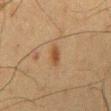Assessment: The lesion was photographed on a routine skin check and not biopsied; there is no pathology result. Clinical summary: The recorded lesion diameter is about 3 mm. The patient is a male about 60 years old. A roughly 15 mm field-of-view crop from a total-body skin photograph. Located on the mid back. Imaged with cross-polarized lighting.